This is a white-light tile; An algorithmic analysis of the crop reported a shape eccentricity near 0.8 and a shape-asymmetry score of about 0.5 (0 = symmetric). The software also gave an average lesion color of about L≈48 a*≈28 b*≈18 (CIELAB), about 10 CIELAB-L* units darker than the surrounding skin, and a normalized lesion–skin contrast near 7.5. It also reported border irregularity of about 4 on a 0–10 scale, a color-variation rating of about 0/10, and peripheral color asymmetry of about 0. The analysis additionally found a classifier nevus-likeness of about 0/100 and lesion-presence confidence of about 100/100; the lesion is on the head or neck; the lesion's longest dimension is about 1 mm; a male patient roughly 45 years of age; a 15 mm close-up tile from a total-body photography series done for melanoma screening:
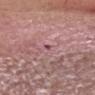On excision, pathology confirmed a superficial basal cell carcinoma (malignant).